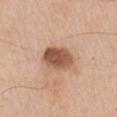biopsy status: catalogued during a skin exam; not biopsied | subject: male, in their mid- to late 60s | image: ~15 mm crop, total-body skin-cancer survey | site: the right upper arm.